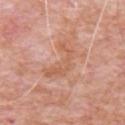{"biopsy_status": "not biopsied; imaged during a skin examination", "automated_metrics": {"area_mm2_approx": 9.5, "eccentricity": 0.85, "vs_skin_darker_L": 7.0, "vs_skin_contrast_norm": 5.5, "border_irregularity_0_10": 8.0, "peripheral_color_asymmetry": 1.0}, "patient": {"sex": "male", "age_approx": 80}, "site": "chest", "image": {"source": "total-body photography crop", "field_of_view_mm": 15}}